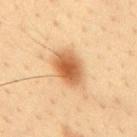<record>
  <biopsy_status>not biopsied; imaged during a skin examination</biopsy_status>
  <image>
    <source>total-body photography crop</source>
    <field_of_view_mm>15</field_of_view_mm>
  </image>
  <patient>
    <sex>male</sex>
    <age_approx>35</age_approx>
  </patient>
  <site>mid back</site>
  <automated_metrics>
    <nevus_likeness_0_100>100</nevus_likeness_0_100>
    <lesion_detection_confidence_0_100>100</lesion_detection_confidence_0_100>
  </automated_metrics>
  <lighting>cross-polarized</lighting>
  <lesion_size>
    <long_diameter_mm_approx>5.0</long_diameter_mm_approx>
  </lesion_size>
</record>